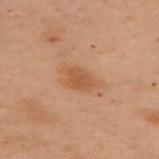This lesion was catalogued during total-body skin photography and was not selected for biopsy.
A 15 mm crop from a total-body photograph taken for skin-cancer surveillance.
On the upper back.
Captured under cross-polarized illumination.
Measured at roughly 3 mm in maximum diameter.
A female subject aged approximately 55.